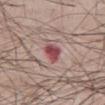lighting: white-light
location: the abdomen
image-analysis metrics: a lesion color around L≈48 a*≈30 b*≈20 in CIELAB, a lesion–skin lightness drop of about 14, and a lesion-to-skin contrast of about 10 (normalized; higher = more distinct); a border-irregularity index near 2.5/10, a within-lesion color-variation index near 3.5/10, and radial color variation of about 1.5; a classifier nevus-likeness of about 0/100
lesion diameter: ~3 mm (longest diameter)
acquisition: ~15 mm crop, total-body skin-cancer survey
patient: male, about 60 years old